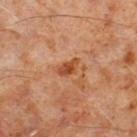Notes:
• biopsy status: imaged on a skin check; not biopsied
• imaging modality: 15 mm crop, total-body photography
• patient: male, about 60 years old
• lighting: cross-polarized illumination
• site: the right lower leg